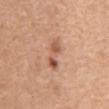lighting = white-light | site = the chest | imaging modality = ~15 mm crop, total-body skin-cancer survey | lesion diameter = about 4 mm | subject = female, aged 53–57 | image-analysis metrics = a lesion area of about 5.5 mm² and an eccentricity of roughly 0.95; an average lesion color of about L≈56 a*≈23 b*≈32 (CIELAB) and about 11 CIELAB-L* units darker than the surrounding skin; a nevus-likeness score of about 70/100 and a lesion-detection confidence of about 100/100.